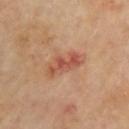The lesion was tiled from a total-body skin photograph and was not biopsied. Located on the right upper arm. A close-up tile cropped from a whole-body skin photograph, about 15 mm across. A male patient aged around 65.